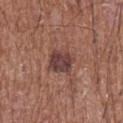The lesion was tiled from a total-body skin photograph and was not biopsied. An algorithmic analysis of the crop reported a lesion color around L≈41 a*≈21 b*≈21 in CIELAB and a normalized border contrast of about 9.5. The software also gave border irregularity of about 2.5 on a 0–10 scale, internal color variation of about 3.5 on a 0–10 scale, and a peripheral color-asymmetry measure near 1. The software also gave a nevus-likeness score of about 5/100 and lesion-presence confidence of about 100/100. The subject is a male aged around 75. About 3 mm across. The tile uses white-light illumination. Cropped from a whole-body photographic skin survey; the tile spans about 15 mm. The lesion is on the chest.